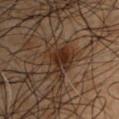Cropped from a total-body skin-imaging series; the visible field is about 15 mm. The recorded lesion diameter is about 5.5 mm. From the left upper arm. A male patient aged approximately 50. Captured under cross-polarized illumination.